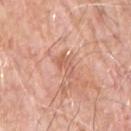Part of a total-body skin-imaging series; this lesion was reviewed on a skin check and was not flagged for biopsy. Located on the chest. The lesion's longest dimension is about 3 mm. Cropped from a whole-body photographic skin survey; the tile spans about 15 mm. Automated image analysis of the tile measured a footprint of about 4 mm², an eccentricity of roughly 0.85, and two-axis asymmetry of about 0.4. It also reported a mean CIELAB color near L≈61 a*≈25 b*≈31 and roughly 8 lightness units darker than nearby skin. And it measured a border-irregularity index near 4.5/10, a within-lesion color-variation index near 1/10, and a peripheral color-asymmetry measure near 0. The software also gave an automated nevus-likeness rating near 0 out of 100 and a lesion-detection confidence of about 100/100. A male patient aged approximately 70.